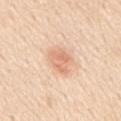diameter = about 3.5 mm
image source = 15 mm crop, total-body photography
subject = male, roughly 60 years of age
image-analysis metrics = a lesion area of about 7.5 mm², a shape eccentricity near 0.7, and two-axis asymmetry of about 0.2; a mean CIELAB color near L≈71 a*≈22 b*≈34, about 10 CIELAB-L* units darker than the surrounding skin, and a normalized border contrast of about 6; an automated nevus-likeness rating near 60 out of 100 and a detector confidence of about 100 out of 100 that the crop contains a lesion
lighting = white-light
site = the mid back